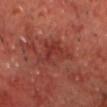Findings:
• workup — no biopsy performed (imaged during a skin exam)
• subject — male, aged approximately 70
• anatomic site — the chest
• tile lighting — cross-polarized illumination
• lesion size — about 5 mm
• automated lesion analysis — a footprint of about 10 mm², an outline eccentricity of about 0.75 (0 = round, 1 = elongated), and a shape-asymmetry score of about 0.5 (0 = symmetric); an average lesion color of about L≈35 a*≈29 b*≈26 (CIELAB), a lesion–skin lightness drop of about 7, and a lesion-to-skin contrast of about 6 (normalized; higher = more distinct); border irregularity of about 5.5 on a 0–10 scale and radial color variation of about 1.5; a lesion-detection confidence of about 100/100
• acquisition — total-body-photography crop, ~15 mm field of view